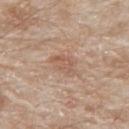Clinical impression:
Captured during whole-body skin photography for melanoma surveillance; the lesion was not biopsied.
Acquisition and patient details:
A male subject, aged 78 to 82. The tile uses white-light illumination. The lesion is located on the upper back. Cropped from a total-body skin-imaging series; the visible field is about 15 mm.